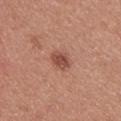Case summary:
– workup — imaged on a skin check; not biopsied
– body site — the upper back
– imaging modality — ~15 mm crop, total-body skin-cancer survey
– tile lighting — white-light
– subject — female, about 25 years old
– lesion diameter — ~2.5 mm (longest diameter)